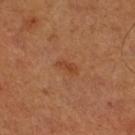<tbp_lesion>
<biopsy_status>not biopsied; imaged during a skin examination</biopsy_status>
<lighting>cross-polarized</lighting>
<patient>
  <sex>male</sex>
  <age_approx>50</age_approx>
</patient>
<site>right thigh</site>
<automated_metrics>
  <area_mm2_approx>3.0</area_mm2_approx>
  <eccentricity>0.85</eccentricity>
  <vs_skin_darker_L>6.0</vs_skin_darker_L>
  <color_variation_0_10>0.5</color_variation_0_10>
  <peripheral_color_asymmetry>0.0</peripheral_color_asymmetry>
  <nevus_likeness_0_100>0</nevus_likeness_0_100>
  <lesion_detection_confidence_0_100>100</lesion_detection_confidence_0_100>
</automated_metrics>
<image>
  <source>total-body photography crop</source>
  <field_of_view_mm>15</field_of_view_mm>
</image>
<lesion_size>
  <long_diameter_mm_approx>2.5</long_diameter_mm_approx>
</lesion_size>
</tbp_lesion>